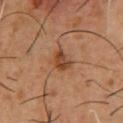Assessment:
No biopsy was performed on this lesion — it was imaged during a full skin examination and was not determined to be concerning.
Acquisition and patient details:
A 15 mm close-up tile from a total-body photography series done for melanoma screening. Located on the chest. The recorded lesion diameter is about 2.5 mm. Captured under cross-polarized illumination. A male subject approximately 55 years of age. The total-body-photography lesion software estimated an eccentricity of roughly 0.65 and two-axis asymmetry of about 0.3. It also reported a mean CIELAB color near L≈42 a*≈22 b*≈34, about 10 CIELAB-L* units darker than the surrounding skin, and a normalized lesion–skin contrast near 8.5. And it measured a border-irregularity index near 2.5/10 and a within-lesion color-variation index near 4.5/10. It also reported a nevus-likeness score of about 65/100.